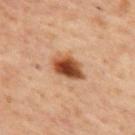biopsy_status: not biopsied; imaged during a skin examination
patient:
  sex: male
  age_approx: 55
image:
  source: total-body photography crop
  field_of_view_mm: 15
lesion_size:
  long_diameter_mm_approx: 4.5
site: back
lighting: cross-polarized